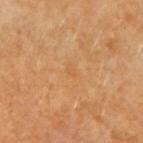Assessment:
Part of a total-body skin-imaging series; this lesion was reviewed on a skin check and was not flagged for biopsy.
Image and clinical context:
The tile uses cross-polarized illumination. A female subject, approximately 50 years of age. Longest diameter approximately 1.5 mm. This image is a 15 mm lesion crop taken from a total-body photograph. From the left forearm.